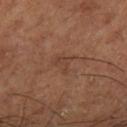follow-up: total-body-photography surveillance lesion; no biopsy | TBP lesion metrics: a footprint of about 4 mm² and a shape-asymmetry score of about 0.55 (0 = symmetric); a classifier nevus-likeness of about 0/100 and lesion-presence confidence of about 100/100 | lesion diameter: about 2.5 mm | illumination: cross-polarized | subject: male, in their mid- to late 60s | imaging modality: ~15 mm crop, total-body skin-cancer survey | location: the left lower leg.